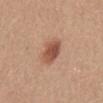Q: What did automated image analysis measure?
A: an area of roughly 7.5 mm², a shape eccentricity near 0.6, and a shape-asymmetry score of about 0.15 (0 = symmetric); roughly 12 lightness units darker than nearby skin and a normalized border contrast of about 8.5; border irregularity of about 1.5 on a 0–10 scale, a color-variation rating of about 4/10, and a peripheral color-asymmetry measure near 1.5
Q: Lesion size?
A: ≈3.5 mm
Q: What is the imaging modality?
A: ~15 mm crop, total-body skin-cancer survey
Q: Illumination type?
A: white-light illumination
Q: Lesion location?
A: the abdomen
Q: What are the patient's age and sex?
A: female, in their mid-50s